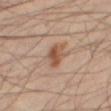Clinical summary:
This is a cross-polarized tile. Cropped from a whole-body photographic skin survey; the tile spans about 15 mm. The lesion is located on the right thigh. The patient is a male aged 43 to 47. About 3.5 mm across.